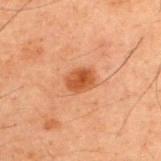notes = no biopsy performed (imaged during a skin exam) | acquisition = ~15 mm tile from a whole-body skin photo | TBP lesion metrics = an area of roughly 5.5 mm², a shape eccentricity near 0.65, and two-axis asymmetry of about 0.2; a border-irregularity index near 2/10, a color-variation rating of about 3/10, and a peripheral color-asymmetry measure near 1 | diameter = ~3 mm (longest diameter) | subject = male, aged 58–62 | site = the upper back.